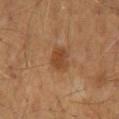Acquisition and patient details: The subject is a male aged approximately 60. Longest diameter approximately 3 mm. The lesion is on the abdomen. Imaged with cross-polarized lighting. A close-up tile cropped from a whole-body skin photograph, about 15 mm across. The total-body-photography lesion software estimated a border-irregularity index near 2/10 and radial color variation of about 0.5. And it measured a classifier nevus-likeness of about 15/100 and a detector confidence of about 100 out of 100 that the crop contains a lesion.